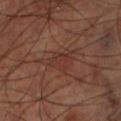The lesion was photographed on a routine skin check and not biopsied; there is no pathology result.
Approximately 5 mm at its widest.
A close-up tile cropped from a whole-body skin photograph, about 15 mm across.
This is a cross-polarized tile.
On the left lower leg.
A male patient, approximately 70 years of age.
Automated tile analysis of the lesion measured a lesion area of about 8 mm², an outline eccentricity of about 0.8 (0 = round, 1 = elongated), and a shape-asymmetry score of about 0.45 (0 = symmetric). It also reported a nevus-likeness score of about 0/100 and a lesion-detection confidence of about 55/100.